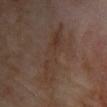Imaged during a routine full-body skin examination; the lesion was not biopsied and no histopathology is available.
A female subject aged around 60.
Measured at roughly 7.5 mm in maximum diameter.
Captured under cross-polarized illumination.
A 15 mm close-up extracted from a 3D total-body photography capture.
Located on the front of the torso.